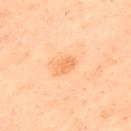No biopsy was performed on this lesion — it was imaged during a full skin examination and was not determined to be concerning. This image is a 15 mm lesion crop taken from a total-body photograph. From the upper back. Captured under cross-polarized illumination. An algorithmic analysis of the crop reported an average lesion color of about L≈72 a*≈26 b*≈45 (CIELAB), a lesion–skin lightness drop of about 8, and a normalized lesion–skin contrast near 6. The analysis additionally found a nevus-likeness score of about 0/100 and a detector confidence of about 100 out of 100 that the crop contains a lesion. A male subject aged around 40.